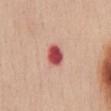The lesion was tiled from a total-body skin photograph and was not biopsied. Approximately 2.5 mm at its widest. A female patient aged 48–52. This is a white-light tile. The total-body-photography lesion software estimated a classifier nevus-likeness of about 0/100 and a detector confidence of about 100 out of 100 that the crop contains a lesion. On the chest. A roughly 15 mm field-of-view crop from a total-body skin photograph.